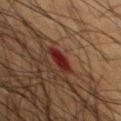Impression: The lesion was photographed on a routine skin check and not biopsied; there is no pathology result. Acquisition and patient details: A male patient, aged 63 to 67. A 15 mm close-up extracted from a 3D total-body photography capture. Captured under cross-polarized illumination. Approximately 3 mm at its widest. The lesion is on the chest. The total-body-photography lesion software estimated a footprint of about 5 mm², an eccentricity of roughly 0.8, and two-axis asymmetry of about 0.25. And it measured an average lesion color of about L≈22 a*≈25 b*≈22 (CIELAB) and a normalized border contrast of about 11. The analysis additionally found a border-irregularity index near 3/10, a within-lesion color-variation index near 3.5/10, and a peripheral color-asymmetry measure near 1.5. And it measured a classifier nevus-likeness of about 0/100 and a detector confidence of about 100 out of 100 that the crop contains a lesion.